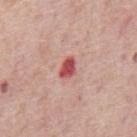Captured during whole-body skin photography for melanoma surveillance; the lesion was not biopsied.